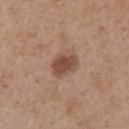{"lesion_size": {"long_diameter_mm_approx": 3.5}, "patient": {"sex": "female", "age_approx": 40}, "image": {"source": "total-body photography crop", "field_of_view_mm": 15}, "lighting": "white-light", "site": "right upper arm"}